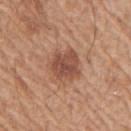No biopsy was performed on this lesion — it was imaged during a full skin examination and was not determined to be concerning. The lesion is on the right upper arm. Captured under white-light illumination. The subject is a male aged 63 to 67. Longest diameter approximately 4 mm. Cropped from a total-body skin-imaging series; the visible field is about 15 mm. The lesion-visualizer software estimated a mean CIELAB color near L≈50 a*≈23 b*≈29, about 11 CIELAB-L* units darker than the surrounding skin, and a normalized border contrast of about 8. The software also gave internal color variation of about 3.5 on a 0–10 scale and a peripheral color-asymmetry measure near 1. The analysis additionally found an automated nevus-likeness rating near 80 out of 100.